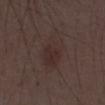| field | value |
|---|---|
| notes | no biopsy performed (imaged during a skin exam) |
| patient | male, aged 48–52 |
| image source | ~15 mm tile from a whole-body skin photo |
| diameter | ~6 mm (longest diameter) |